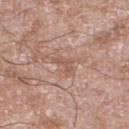Imaged during a routine full-body skin examination; the lesion was not biopsied and no histopathology is available.
A male subject, aged around 60.
The lesion is located on the leg.
A lesion tile, about 15 mm wide, cut from a 3D total-body photograph.